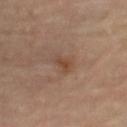{"biopsy_status": "not biopsied; imaged during a skin examination", "automated_metrics": {"area_mm2_approx": 3.5, "eccentricity": 0.75, "shape_asymmetry": 0.4, "cielab_L": 46, "cielab_a": 19, "cielab_b": 30, "vs_skin_darker_L": 7.0, "vs_skin_contrast_norm": 6.5, "border_irregularity_0_10": 4.0, "color_variation_0_10": 2.0, "peripheral_color_asymmetry": 0.5, "nevus_likeness_0_100": 15, "lesion_detection_confidence_0_100": 100}, "image": {"source": "total-body photography crop", "field_of_view_mm": 15}, "lesion_size": {"long_diameter_mm_approx": 2.5}, "patient": {"sex": "female", "age_approx": 65}, "site": "right lower leg", "lighting": "cross-polarized"}